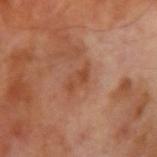Impression:
The lesion was photographed on a routine skin check and not biopsied; there is no pathology result.
Image and clinical context:
Longest diameter approximately 3.5 mm. Automated tile analysis of the lesion measured roughly 7 lightness units darker than nearby skin and a normalized lesion–skin contrast near 6.5. It also reported a border-irregularity index near 4.5/10, a color-variation rating of about 2.5/10, and peripheral color asymmetry of about 0.5. The analysis additionally found an automated nevus-likeness rating near 0 out of 100 and lesion-presence confidence of about 100/100. A roughly 15 mm field-of-view crop from a total-body skin photograph. On the right upper arm. A male patient, roughly 70 years of age. This is a cross-polarized tile.